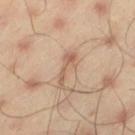size — ≈4.5 mm
imaging modality — 15 mm crop, total-body photography
patient — male, aged 43 to 47
automated metrics — a lesion–skin lightness drop of about 9 and a lesion-to-skin contrast of about 6 (normalized; higher = more distinct); border irregularity of about 6 on a 0–10 scale and a within-lesion color-variation index near 3.5/10
body site — the left thigh
lighting — cross-polarized illumination